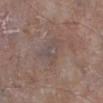Q: Who is the patient?
A: male, aged approximately 85 or older
Q: What is the lesion's diameter?
A: ≈5 mm
Q: How was this image acquired?
A: total-body-photography crop, ~15 mm field of view
Q: What lighting was used for the tile?
A: white-light illumination
Q: What is the anatomic site?
A: the right lower leg